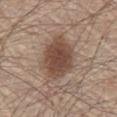Q: Was a biopsy performed?
A: imaged on a skin check; not biopsied
Q: Patient demographics?
A: male, approximately 80 years of age
Q: Lesion size?
A: ≈5.5 mm
Q: Where on the body is the lesion?
A: the front of the torso
Q: What is the imaging modality?
A: total-body-photography crop, ~15 mm field of view
Q: Illumination type?
A: white-light illumination